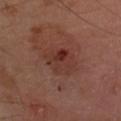Q: Was a biopsy performed?
A: total-body-photography surveillance lesion; no biopsy